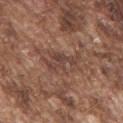{"biopsy_status": "not biopsied; imaged during a skin examination", "image": {"source": "total-body photography crop", "field_of_view_mm": 15}, "site": "upper back", "lesion_size": {"long_diameter_mm_approx": 4.5}, "lighting": "white-light", "patient": {"sex": "male", "age_approx": 75}}